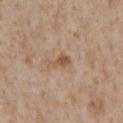Q: Is there a histopathology result?
A: total-body-photography surveillance lesion; no biopsy
Q: How large is the lesion?
A: ~2.5 mm (longest diameter)
Q: What kind of image is this?
A: ~15 mm crop, total-body skin-cancer survey
Q: Where on the body is the lesion?
A: the chest
Q: Patient demographics?
A: male, aged 63–67